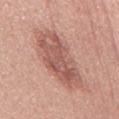Case summary:
• notes · catalogued during a skin exam; not biopsied
• tile lighting · white-light illumination
• anatomic site · the chest
• image-analysis metrics · an area of roughly 24 mm², an outline eccentricity of about 0.95 (0 = round, 1 = elongated), and a shape-asymmetry score of about 0.2 (0 = symmetric); an automated nevus-likeness rating near 10 out of 100 and lesion-presence confidence of about 100/100
• acquisition · total-body-photography crop, ~15 mm field of view
• patient · female, aged approximately 45
• lesion size · about 9 mm